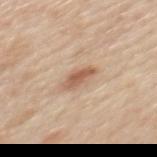Findings:
- workup — no biopsy performed (imaged during a skin exam)
- automated lesion analysis — a shape eccentricity near 0.9 and a symmetry-axis asymmetry near 0.3; a classifier nevus-likeness of about 60/100 and a detector confidence of about 100 out of 100 that the crop contains a lesion
- lighting — white-light
- body site — the mid back
- diameter — about 3.5 mm
- subject — male, aged 58 to 62
- image — total-body-photography crop, ~15 mm field of view